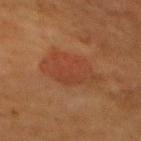follow-up: imaged on a skin check; not biopsied | location: the mid back | TBP lesion metrics: an area of roughly 18 mm², an eccentricity of roughly 0.85, and a shape-asymmetry score of about 0.25 (0 = symmetric) | imaging modality: 15 mm crop, total-body photography | size: about 6.5 mm | patient: female, aged approximately 50.